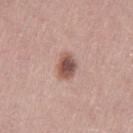Q: Is there a histopathology result?
A: catalogued during a skin exam; not biopsied
Q: Lesion size?
A: ~3.5 mm (longest diameter)
Q: Illumination type?
A: white-light illumination
Q: What kind of image is this?
A: ~15 mm tile from a whole-body skin photo
Q: Where on the body is the lesion?
A: the leg
Q: Patient demographics?
A: female, aged approximately 50